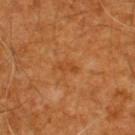Measured at roughly 2.5 mm in maximum diameter.
A male patient, aged 58–62.
A roughly 15 mm field-of-view crop from a total-body skin photograph.
From the upper back.
Captured under cross-polarized illumination.